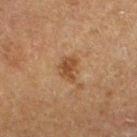Part of a total-body skin-imaging series; this lesion was reviewed on a skin check and was not flagged for biopsy.
Cropped from a total-body skin-imaging series; the visible field is about 15 mm.
A female patient, aged around 50.
Automated image analysis of the tile measured an outline eccentricity of about 0.7 (0 = round, 1 = elongated) and a shape-asymmetry score of about 0.35 (0 = symmetric). The analysis additionally found a lesion color around L≈38 a*≈18 b*≈30 in CIELAB, a lesion–skin lightness drop of about 8, and a normalized border contrast of about 7.5.
The tile uses cross-polarized illumination.
About 3 mm across.
The lesion is on the arm.